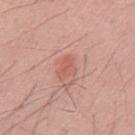Recorded during total-body skin imaging; not selected for excision or biopsy. The recorded lesion diameter is about 3 mm. The lesion is located on the mid back. A 15 mm close-up extracted from a 3D total-body photography capture. A male patient about 30 years old. Captured under white-light illumination.